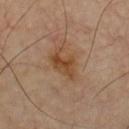Q: Was this lesion biopsied?
A: imaged on a skin check; not biopsied
Q: Lesion location?
A: the front of the torso
Q: Illumination type?
A: cross-polarized
Q: How was this image acquired?
A: total-body-photography crop, ~15 mm field of view
Q: What are the patient's age and sex?
A: male, in their 60s